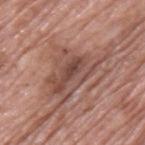No biopsy was performed on this lesion — it was imaged during a full skin examination and was not determined to be concerning.